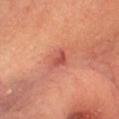Q: Was a biopsy performed?
A: imaged on a skin check; not biopsied
Q: What kind of image is this?
A: total-body-photography crop, ~15 mm field of view
Q: What is the anatomic site?
A: the head or neck
Q: Patient demographics?
A: female, in their mid-60s
Q: How was the tile lit?
A: cross-polarized illumination
Q: Lesion size?
A: about 3.5 mm